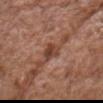The lesion was photographed on a routine skin check and not biopsied; there is no pathology result.
Longest diameter approximately 3.5 mm.
Located on the head or neck.
A 15 mm crop from a total-body photograph taken for skin-cancer surveillance.
A male subject aged 73 to 77.
An algorithmic analysis of the crop reported an area of roughly 5 mm². And it measured a mean CIELAB color near L≈43 a*≈22 b*≈28 and roughly 11 lightness units darker than nearby skin. The software also gave a border-irregularity rating of about 3/10, internal color variation of about 4.5 on a 0–10 scale, and radial color variation of about 1.5. And it measured a lesion-detection confidence of about 100/100.
This is a white-light tile.